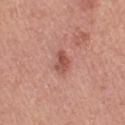Q: Is there a histopathology result?
A: imaged on a skin check; not biopsied
Q: What are the patient's age and sex?
A: female, aged 53–57
Q: Where on the body is the lesion?
A: the leg
Q: How was this image acquired?
A: ~15 mm tile from a whole-body skin photo